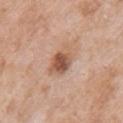The lesion was photographed on a routine skin check and not biopsied; there is no pathology result. This is a white-light tile. A 15 mm close-up tile from a total-body photography series done for melanoma screening. The lesion is on the left upper arm. The patient is a female aged 68–72. The lesion-visualizer software estimated a footprint of about 8.5 mm² and an eccentricity of roughly 0.65.